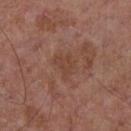Case summary:
* workup: total-body-photography surveillance lesion; no biopsy
* acquisition: ~15 mm crop, total-body skin-cancer survey
* lesion size: ≈3 mm
* site: the chest
* subject: male, aged around 55
* automated lesion analysis: a lesion color around L≈43 a*≈20 b*≈28 in CIELAB and about 6 CIELAB-L* units darker than the surrounding skin; a detector confidence of about 100 out of 100 that the crop contains a lesion